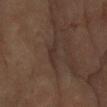Context: Measured at roughly 3 mm in maximum diameter. The total-body-photography lesion software estimated a lesion area of about 3.5 mm², a shape eccentricity near 0.85, and a symmetry-axis asymmetry near 0.45. Cropped from a whole-body photographic skin survey; the tile spans about 15 mm. The lesion is located on the right forearm. A female patient, aged around 70.